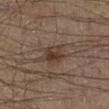Captured during whole-body skin photography for melanoma surveillance; the lesion was not biopsied. Measured at roughly 2.5 mm in maximum diameter. The lesion is located on the right lower leg. This is a cross-polarized tile. A region of skin cropped from a whole-body photographic capture, roughly 15 mm wide. A male subject, roughly 65 years of age.